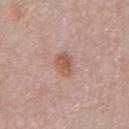Notes:
• image — ~15 mm tile from a whole-body skin photo
• location — the front of the torso
• lighting — white-light illumination
• subject — male, in their mid-50s
• lesion diameter — about 2.5 mm